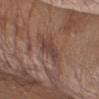{"biopsy_status": "not biopsied; imaged during a skin examination"}